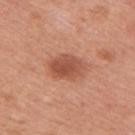- lighting: white-light
- imaging modality: ~15 mm tile from a whole-body skin photo
- TBP lesion metrics: an average lesion color of about L≈52 a*≈27 b*≈32 (CIELAB), roughly 11 lightness units darker than nearby skin, and a normalized border contrast of about 7.5
- subject: female, aged around 50
- location: the right upper arm